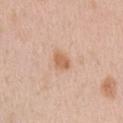Q: Was a biopsy performed?
A: total-body-photography surveillance lesion; no biopsy
Q: How was this image acquired?
A: ~15 mm crop, total-body skin-cancer survey
Q: What are the patient's age and sex?
A: male, aged around 50
Q: How was the tile lit?
A: white-light
Q: Lesion size?
A: ~2.5 mm (longest diameter)
Q: What did automated image analysis measure?
A: a lesion area of about 3.5 mm², a shape eccentricity near 0.8, and a symmetry-axis asymmetry near 0.3; a lesion color around L≈62 a*≈22 b*≈34 in CIELAB, a lesion–skin lightness drop of about 10, and a normalized lesion–skin contrast near 7.5; an automated nevus-likeness rating near 55 out of 100 and lesion-presence confidence of about 100/100
Q: What is the anatomic site?
A: the right upper arm